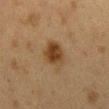Findings:
• workup · catalogued during a skin exam; not biopsied
• acquisition · total-body-photography crop, ~15 mm field of view
• location · the mid back
• subject · female, approximately 40 years of age
• lesion diameter · ≈4 mm
• lighting · cross-polarized illumination